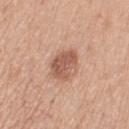Acquisition and patient details:
The lesion is on the arm. The lesion-visualizer software estimated border irregularity of about 2.5 on a 0–10 scale. The tile uses white-light illumination. A female subject aged around 55. A 15 mm close-up tile from a total-body photography series done for melanoma screening.